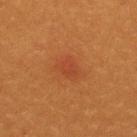The lesion was photographed on a routine skin check and not biopsied; there is no pathology result. This is a cross-polarized tile. Approximately 2.5 mm at its widest. The lesion is on the upper back. Automated tile analysis of the lesion measured a lesion–skin lightness drop of about 5. It also reported border irregularity of about 3 on a 0–10 scale, a within-lesion color-variation index near 2.5/10, and peripheral color asymmetry of about 1. A 15 mm close-up extracted from a 3D total-body photography capture. The patient is a female roughly 30 years of age.